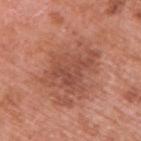Background: Imaged with white-light lighting. A male patient, approximately 70 years of age. A roughly 15 mm field-of-view crop from a total-body skin photograph. The recorded lesion diameter is about 6.5 mm. The lesion is located on the upper back.